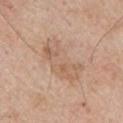Case summary:
* workup: imaged on a skin check; not biopsied
* location: the left upper arm
* subject: male, about 60 years old
* acquisition: ~15 mm tile from a whole-body skin photo
* lesion diameter: about 6 mm